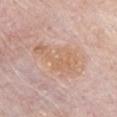Assessment:
Imaged during a routine full-body skin examination; the lesion was not biopsied and no histopathology is available.
Clinical summary:
The patient is a male aged 73–77. The lesion is on the chest. Imaged with white-light lighting. A roughly 15 mm field-of-view crop from a total-body skin photograph. Measured at roughly 6 mm in maximum diameter.